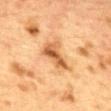patient = female, about 40 years old | lighting = cross-polarized illumination | image source = ~15 mm tile from a whole-body skin photo | image-analysis metrics = a border-irregularity rating of about 5/10, a color-variation rating of about 6.5/10, and peripheral color asymmetry of about 2.5 | anatomic site = the mid back | diameter = ~4.5 mm (longest diameter).